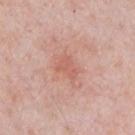No biopsy was performed on this lesion — it was imaged during a full skin examination and was not determined to be concerning.
A male subject roughly 60 years of age.
The lesion's longest dimension is about 2.5 mm.
A 15 mm crop from a total-body photograph taken for skin-cancer surveillance.
The lesion is located on the front of the torso.